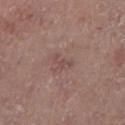Q: Was this lesion biopsied?
A: no biopsy performed (imaged during a skin exam)
Q: Lesion size?
A: ≈2.5 mm
Q: Patient demographics?
A: male, in their 80s
Q: Automated lesion metrics?
A: a lesion area of about 2.5 mm² and two-axis asymmetry of about 0.55; roughly 6 lightness units darker than nearby skin and a normalized lesion–skin contrast near 5; a border-irregularity index near 7/10; a nevus-likeness score of about 0/100
Q: How was this image acquired?
A: ~15 mm tile from a whole-body skin photo
Q: Where on the body is the lesion?
A: the right lower leg
Q: What lighting was used for the tile?
A: white-light